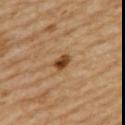follow-up: total-body-photography surveillance lesion; no biopsy
TBP lesion metrics: a footprint of about 3.5 mm² and an outline eccentricity of about 0.75 (0 = round, 1 = elongated); an average lesion color of about L≈45 a*≈21 b*≈37 (CIELAB), about 14 CIELAB-L* units darker than the surrounding skin, and a normalized border contrast of about 10.5; a border-irregularity index near 2/10, a color-variation rating of about 5/10, and radial color variation of about 1.5; a classifier nevus-likeness of about 100/100
patient: female, aged 53–57
site: the upper back
lesion diameter: about 2.5 mm
imaging modality: 15 mm crop, total-body photography